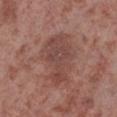biopsy_status: not biopsied; imaged during a skin examination
patient:
  sex: male
  age_approx: 55
site: left lower leg
image:
  source: total-body photography crop
  field_of_view_mm: 15